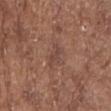Part of a total-body skin-imaging series; this lesion was reviewed on a skin check and was not flagged for biopsy. Imaged with white-light lighting. Measured at roughly 3.5 mm in maximum diameter. The lesion is on the left forearm. Automated tile analysis of the lesion measured a footprint of about 4 mm² and an outline eccentricity of about 0.9 (0 = round, 1 = elongated). The analysis additionally found a mean CIELAB color near L≈44 a*≈20 b*≈24, about 6 CIELAB-L* units darker than the surrounding skin, and a normalized lesion–skin contrast near 5. It also reported a detector confidence of about 95 out of 100 that the crop contains a lesion. A female patient, in their mid- to late 70s. This image is a 15 mm lesion crop taken from a total-body photograph.